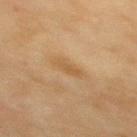  biopsy_status: not biopsied; imaged during a skin examination
  lesion_size:
    long_diameter_mm_approx: 3.0
  image:
    source: total-body photography crop
    field_of_view_mm: 15
  site: upper back
  lighting: cross-polarized
  patient:
    sex: female
    age_approx: 55